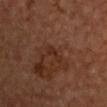<lesion>
  <biopsy_status>not biopsied; imaged during a skin examination</biopsy_status>
  <lighting>cross-polarized</lighting>
  <patient>
    <sex>male</sex>
    <age_approx>70</age_approx>
  </patient>
  <site>chest</site>
  <image>
    <source>total-body photography crop</source>
    <field_of_view_mm>15</field_of_view_mm>
  </image>
  <lesion_size>
    <long_diameter_mm_approx>2.5</long_diameter_mm_approx>
  </lesion_size>
</lesion>